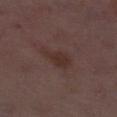<tbp_lesion>
  <biopsy_status>not biopsied; imaged during a skin examination</biopsy_status>
  <lesion_size>
    <long_diameter_mm_approx>4.5</long_diameter_mm_approx>
  </lesion_size>
  <lighting>white-light</lighting>
  <site>left lower leg</site>
  <image>
    <source>total-body photography crop</source>
    <field_of_view_mm>15</field_of_view_mm>
  </image>
  <patient>
    <sex>female</sex>
    <age_approx>30</age_approx>
  </patient>
  <automated_metrics>
    <area_mm2_approx>7.0</area_mm2_approx>
    <eccentricity>0.85</eccentricity>
    <shape_asymmetry>0.4</shape_asymmetry>
    <border_irregularity_0_10>4.0</border_irregularity_0_10>
    <color_variation_0_10>1.5</color_variation_0_10>
  </automated_metrics>
</tbp_lesion>